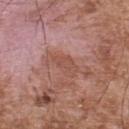The lesion was photographed on a routine skin check and not biopsied; there is no pathology result.
On the upper back.
A roughly 15 mm field-of-view crop from a total-body skin photograph.
A male patient roughly 55 years of age.